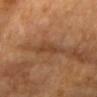Captured during whole-body skin photography for melanoma surveillance; the lesion was not biopsied.